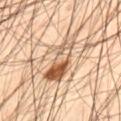<record>
  <biopsy_status>not biopsied; imaged during a skin examination</biopsy_status>
  <lesion_size>
    <long_diameter_mm_approx>7.0</long_diameter_mm_approx>
  </lesion_size>
  <automated_metrics>
    <cielab_L>58</cielab_L>
    <cielab_a>18</cielab_a>
    <cielab_b>33</cielab_b>
    <vs_skin_darker_L>13.0</vs_skin_darker_L>
    <vs_skin_contrast_norm>8.5</vs_skin_contrast_norm>
    <nevus_likeness_0_100>95</nevus_likeness_0_100>
    <lesion_detection_confidence_0_100>95</lesion_detection_confidence_0_100>
  </automated_metrics>
  <patient>
    <sex>male</sex>
    <age_approx>45</age_approx>
  </patient>
  <site>left thigh</site>
  <lighting>cross-polarized</lighting>
  <image>
    <source>total-body photography crop</source>
    <field_of_view_mm>15</field_of_view_mm>
  </image>
</record>